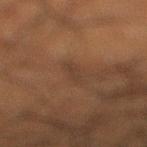Q: Is there a histopathology result?
A: catalogued during a skin exam; not biopsied
Q: What kind of image is this?
A: ~15 mm tile from a whole-body skin photo
Q: Who is the patient?
A: male, in their 50s
Q: What is the lesion's diameter?
A: ~2.5 mm (longest diameter)
Q: Automated lesion metrics?
A: a lesion color around L≈29 a*≈14 b*≈22 in CIELAB and a lesion-to-skin contrast of about 5 (normalized; higher = more distinct); a border-irregularity index near 4.5/10 and peripheral color asymmetry of about 0; a detector confidence of about 65 out of 100 that the crop contains a lesion
Q: What lighting was used for the tile?
A: cross-polarized illumination
Q: What is the anatomic site?
A: the right lower leg